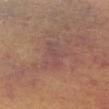Captured during whole-body skin photography for melanoma surveillance; the lesion was not biopsied.
Cropped from a total-body skin-imaging series; the visible field is about 15 mm.
A female patient aged around 50.
From the front of the torso.
Longest diameter approximately 3.5 mm.